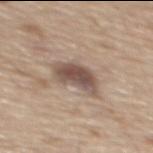{"biopsy_status": "not biopsied; imaged during a skin examination", "automated_metrics": {"area_mm2_approx": 10.0, "eccentricity": 0.8, "shape_asymmetry": 0.25, "color_variation_0_10": 4.5}, "patient": {"sex": "male", "age_approx": 70}, "image": {"source": "total-body photography crop", "field_of_view_mm": 15}, "lighting": "white-light", "site": "mid back", "lesion_size": {"long_diameter_mm_approx": 4.5}}